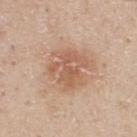This lesion was catalogued during total-body skin photography and was not selected for biopsy. On the upper back. A male subject, aged around 30. Imaged with white-light lighting. A close-up tile cropped from a whole-body skin photograph, about 15 mm across.